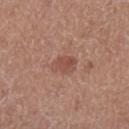biopsy status: no biopsy performed (imaged during a skin exam)
location: the left lower leg
image: total-body-photography crop, ~15 mm field of view
lesion diameter: ≈3 mm
illumination: white-light
patient: male, aged approximately 50